A lesion tile, about 15 mm wide, cut from a 3D total-body photograph. The subject is a male in their mid-50s. Located on the arm:
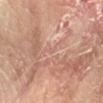On excision, pathology confirmed a squamous cell carcinoma in situ, classified as a malignant skin lesion.